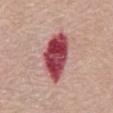Assessment: Part of a total-body skin-imaging series; this lesion was reviewed on a skin check and was not flagged for biopsy. Image and clinical context: A 15 mm close-up extracted from a 3D total-body photography capture. The lesion-visualizer software estimated an area of roughly 18 mm², a shape eccentricity near 0.85, and a shape-asymmetry score of about 0.2 (0 = symmetric). It also reported a mean CIELAB color near L≈47 a*≈35 b*≈20, about 21 CIELAB-L* units darker than the surrounding skin, and a lesion-to-skin contrast of about 14 (normalized; higher = more distinct). It also reported a peripheral color-asymmetry measure near 2. The lesion is on the front of the torso. Captured under white-light illumination. A male subject, aged around 80. Longest diameter approximately 6.5 mm.